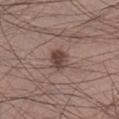Findings:
* notes — imaged on a skin check; not biopsied
* lesion diameter — about 2.5 mm
* automated lesion analysis — a lesion color around L≈43 a*≈18 b*≈22 in CIELAB and about 11 CIELAB-L* units darker than the surrounding skin; border irregularity of about 1.5 on a 0–10 scale and radial color variation of about 1; a classifier nevus-likeness of about 75/100
* body site — the right lower leg
* image source — ~15 mm tile from a whole-body skin photo
* patient — male, approximately 30 years of age